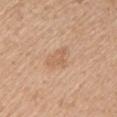Assessment:
The lesion was photographed on a routine skin check and not biopsied; there is no pathology result.
Background:
A female subject, aged 48–52. Cropped from a total-body skin-imaging series; the visible field is about 15 mm. The recorded lesion diameter is about 3 mm. The lesion is on the left upper arm. The total-body-photography lesion software estimated a border-irregularity rating of about 4/10, internal color variation of about 1.5 on a 0–10 scale, and a peripheral color-asymmetry measure near 0.5. The tile uses white-light illumination.